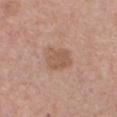Acquisition and patient details:
Captured under white-light illumination. Automated image analysis of the tile measured about 8 CIELAB-L* units darker than the surrounding skin. And it measured an automated nevus-likeness rating near 5 out of 100 and lesion-presence confidence of about 100/100. A roughly 15 mm field-of-view crop from a total-body skin photograph. Located on the chest. The patient is a female in their mid-40s. About 3.5 mm across.